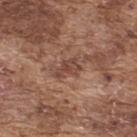Captured during whole-body skin photography for melanoma surveillance; the lesion was not biopsied.
A male patient aged 73–77.
A lesion tile, about 15 mm wide, cut from a 3D total-body photograph.
The lesion is on the back.
This is a white-light tile.
Longest diameter approximately 3 mm.